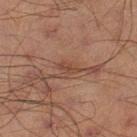Part of a total-body skin-imaging series; this lesion was reviewed on a skin check and was not flagged for biopsy. The lesion's longest dimension is about 2.5 mm. Automated tile analysis of the lesion measured a lesion color around L≈35 a*≈18 b*≈24 in CIELAB, about 6 CIELAB-L* units darker than the surrounding skin, and a normalized border contrast of about 6. The software also gave border irregularity of about 4 on a 0–10 scale. A roughly 15 mm field-of-view crop from a total-body skin photograph. The lesion is located on the left lower leg. A male patient aged 68 to 72.